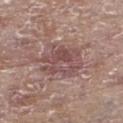This lesion was catalogued during total-body skin photography and was not selected for biopsy. A 15 mm close-up extracted from a 3D total-body photography capture. About 5 mm across. The lesion-visualizer software estimated a lesion area of about 16 mm² and an eccentricity of roughly 0.65. The software also gave roughly 9 lightness units darker than nearby skin and a normalized border contrast of about 6.5. And it measured a border-irregularity index near 5/10, a within-lesion color-variation index near 5.5/10, and a peripheral color-asymmetry measure near 2. It also reported a classifier nevus-likeness of about 0/100 and lesion-presence confidence of about 90/100. The subject is a female aged 83 or over. Captured under white-light illumination. The lesion is located on the left lower leg.